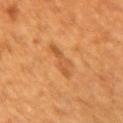Impression:
Part of a total-body skin-imaging series; this lesion was reviewed on a skin check and was not flagged for biopsy.
Background:
The tile uses cross-polarized illumination. About 4.5 mm across. Automated image analysis of the tile measured a lesion area of about 5.5 mm² and an outline eccentricity of about 0.9 (0 = round, 1 = elongated). The software also gave an average lesion color of about L≈46 a*≈22 b*≈37 (CIELAB), roughly 7 lightness units darker than nearby skin, and a normalized lesion–skin contrast near 5.5. The lesion is on the right upper arm. A male subject about 60 years old. A roughly 15 mm field-of-view crop from a total-body skin photograph.